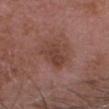{
  "biopsy_status": "not biopsied; imaged during a skin examination",
  "patient": {
    "sex": "male",
    "age_approx": 50
  },
  "lighting": "white-light",
  "lesion_size": {
    "long_diameter_mm_approx": 4.0
  },
  "image": {
    "source": "total-body photography crop",
    "field_of_view_mm": 15
  },
  "site": "head or neck",
  "automated_metrics": {
    "area_mm2_approx": 8.5,
    "eccentricity": 0.8,
    "cielab_L": 41,
    "cielab_a": 21,
    "cielab_b": 24,
    "vs_skin_darker_L": 8.0,
    "vs_skin_contrast_norm": 6.5,
    "border_irregularity_0_10": 3.0,
    "color_variation_0_10": 3.0,
    "peripheral_color_asymmetry": 1.0,
    "nevus_likeness_0_100": 0,
    "lesion_detection_confidence_0_100": 100
  }
}